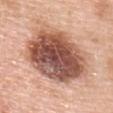Findings:
- follow-up · catalogued during a skin exam; not biopsied
- image source · total-body-photography crop, ~15 mm field of view
- lighting · white-light
- TBP lesion metrics · a border-irregularity rating of about 2/10, internal color variation of about 9.5 on a 0–10 scale, and a peripheral color-asymmetry measure near 3
- anatomic site · the upper back
- size · ≈9.5 mm
- subject · female, about 50 years old